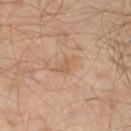A roughly 15 mm field-of-view crop from a total-body skin photograph.
This is a cross-polarized tile.
Located on the left leg.
An algorithmic analysis of the crop reported a lesion color around L≈56 a*≈19 b*≈33 in CIELAB, a lesion–skin lightness drop of about 6, and a lesion-to-skin contrast of about 5 (normalized; higher = more distinct). And it measured a border-irregularity index near 5/10, a color-variation rating of about 0/10, and radial color variation of about 0. It also reported an automated nevus-likeness rating near 0 out of 100 and lesion-presence confidence of about 100/100.
A male patient aged 28 to 32.
Approximately 2.5 mm at its widest.